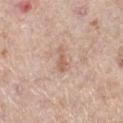– workup · total-body-photography surveillance lesion; no biopsy
– subject · female, approximately 65 years of age
– lesion diameter · ≈3 mm
– location · the left lower leg
– lighting · white-light
– automated lesion analysis · a footprint of about 3 mm², an outline eccentricity of about 0.9 (0 = round, 1 = elongated), and a symmetry-axis asymmetry near 0.35; a mean CIELAB color near L≈60 a*≈20 b*≈28, a lesion–skin lightness drop of about 9, and a normalized border contrast of about 6; internal color variation of about 0.5 on a 0–10 scale and a peripheral color-asymmetry measure near 0
– image source · total-body-photography crop, ~15 mm field of view